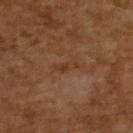Context: From the upper back. A lesion tile, about 15 mm wide, cut from a 3D total-body photograph. The tile uses cross-polarized illumination. The subject is a male about 60 years old.